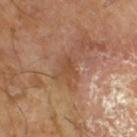Q: Was this lesion biopsied?
A: imaged on a skin check; not biopsied
Q: How large is the lesion?
A: about 3 mm
Q: What lighting was used for the tile?
A: cross-polarized
Q: What did automated image analysis measure?
A: a lesion area of about 4 mm² and an eccentricity of roughly 0.75; a lesion color around L≈46 a*≈21 b*≈32 in CIELAB, about 7 CIELAB-L* units darker than the surrounding skin, and a normalized lesion–skin contrast near 6; a color-variation rating of about 1.5/10 and radial color variation of about 0.5; a classifier nevus-likeness of about 0/100 and a detector confidence of about 100 out of 100 that the crop contains a lesion
Q: What are the patient's age and sex?
A: male, in their mid-60s
Q: How was this image acquired?
A: 15 mm crop, total-body photography